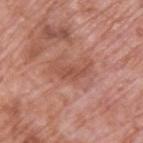Assessment:
Part of a total-body skin-imaging series; this lesion was reviewed on a skin check and was not flagged for biopsy.
Background:
The tile uses white-light illumination. A male subject roughly 70 years of age. On the upper back. The lesion's longest dimension is about 4.5 mm. This image is a 15 mm lesion crop taken from a total-body photograph.